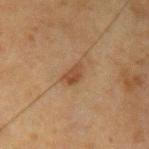Case summary:
- notes — catalogued during a skin exam; not biopsied
- patient — male, aged 48–52
- TBP lesion metrics — a lesion area of about 5 mm², an eccentricity of roughly 0.8, and a shape-asymmetry score of about 0.25 (0 = symmetric); a classifier nevus-likeness of about 70/100 and lesion-presence confidence of about 100/100
- acquisition — 15 mm crop, total-body photography
- location — the left upper arm